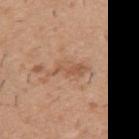This lesion was catalogued during total-body skin photography and was not selected for biopsy. The lesion is on the left upper arm. A 15 mm crop from a total-body photograph taken for skin-cancer surveillance. A male subject, aged 38–42.